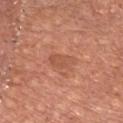biopsy status=imaged on a skin check; not biopsied
location=the chest
lesion size=about 3.5 mm
lighting=white-light illumination
image source=~15 mm tile from a whole-body skin photo
patient=male, approximately 65 years of age